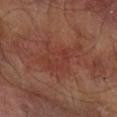{"biopsy_status": "not biopsied; imaged during a skin examination", "patient": {"sex": "male", "age_approx": 70}, "image": {"source": "total-body photography crop", "field_of_view_mm": 15}, "site": "right forearm", "lesion_size": {"long_diameter_mm_approx": 6.0}}